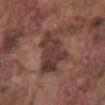On the abdomen. A male patient, aged 73 to 77. A lesion tile, about 15 mm wide, cut from a 3D total-body photograph.